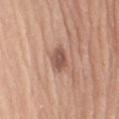No biopsy was performed on this lesion — it was imaged during a full skin examination and was not determined to be concerning. A female subject, aged around 75. This is a white-light tile. Located on the arm. About 3 mm across. This image is a 15 mm lesion crop taken from a total-body photograph. The lesion-visualizer software estimated a footprint of about 6 mm². And it measured a classifier nevus-likeness of about 30/100.